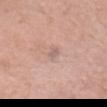Recorded during total-body skin imaging; not selected for excision or biopsy. A close-up tile cropped from a whole-body skin photograph, about 15 mm across. A female subject aged around 55. The lesion is located on the head or neck. Measured at roughly 1.5 mm in maximum diameter.